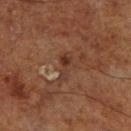location: the right lower leg; image source: ~15 mm tile from a whole-body skin photo; subject: male, about 70 years old.